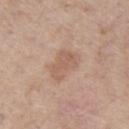Notes:
• biopsy status — catalogued during a skin exam; not biopsied
• image — 15 mm crop, total-body photography
• subject — male, in their 60s
• body site — the left thigh
• tile lighting — white-light illumination
• image-analysis metrics — a lesion area of about 9.5 mm², a shape eccentricity near 0.75, and a symmetry-axis asymmetry near 0.25; a mean CIELAB color near L≈59 a*≈19 b*≈28, roughly 7 lightness units darker than nearby skin, and a normalized lesion–skin contrast near 5; a border-irregularity index near 2.5/10, internal color variation of about 2 on a 0–10 scale, and a peripheral color-asymmetry measure near 1; a nevus-likeness score of about 5/100 and a lesion-detection confidence of about 100/100
• diameter — ≈4 mm